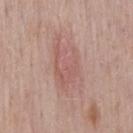The lesion was tiled from a total-body skin photograph and was not biopsied. A male subject aged 53 to 57. The recorded lesion diameter is about 7 mm. A lesion tile, about 15 mm wide, cut from a 3D total-body photograph. Captured under white-light illumination. The lesion is on the back.